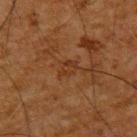Imaged during a routine full-body skin examination; the lesion was not biopsied and no histopathology is available. Automated tile analysis of the lesion measured a lesion area of about 3.5 mm², a shape eccentricity near 0.75, and a symmetry-axis asymmetry near 0.3. And it measured an automated nevus-likeness rating near 0 out of 100 and lesion-presence confidence of about 100/100. A 15 mm crop from a total-body photograph taken for skin-cancer surveillance. From the upper back. The subject is a male about 65 years old. The tile uses cross-polarized illumination.